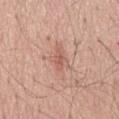follow-up = catalogued during a skin exam; not biopsied
patient = male, aged 63 to 67
lighting = white-light
site = the mid back
acquisition = 15 mm crop, total-body photography